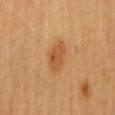workup = no biopsy performed (imaged during a skin exam) | patient = female, roughly 60 years of age | illumination = cross-polarized | location = the mid back | lesion size = ≈3.5 mm | acquisition = total-body-photography crop, ~15 mm field of view.